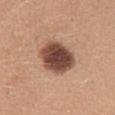– follow-up: no biopsy performed (imaged during a skin exam)
– lesion size: ≈5 mm
– imaging modality: ~15 mm crop, total-body skin-cancer survey
– anatomic site: the back
– TBP lesion metrics: a lesion area of about 16 mm² and an outline eccentricity of about 0.5 (0 = round, 1 = elongated); a lesion color around L≈46 a*≈21 b*≈26 in CIELAB and a lesion-to-skin contrast of about 13.5 (normalized; higher = more distinct); a classifier nevus-likeness of about 40/100 and a lesion-detection confidence of about 100/100
– subject: female, about 30 years old